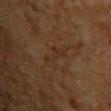workup: imaged on a skin check; not biopsied | diameter: ≈4 mm | patient: male, in their mid- to late 60s | acquisition: ~15 mm tile from a whole-body skin photo | tile lighting: cross-polarized | body site: the chest.